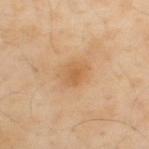Clinical impression:
The lesion was photographed on a routine skin check and not biopsied; there is no pathology result.
Acquisition and patient details:
Longest diameter approximately 3 mm. On the back. This is a cross-polarized tile. The total-body-photography lesion software estimated a footprint of about 5 mm², an outline eccentricity of about 0.7 (0 = round, 1 = elongated), and a symmetry-axis asymmetry near 0.15. The analysis additionally found a mean CIELAB color near L≈60 a*≈20 b*≈40, about 7 CIELAB-L* units darker than the surrounding skin, and a normalized border contrast of about 5.5. The analysis additionally found an automated nevus-likeness rating near 15 out of 100. A male subject aged 53 to 57. A roughly 15 mm field-of-view crop from a total-body skin photograph.